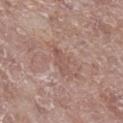  biopsy_status: not biopsied; imaged during a skin examination
  image:
    source: total-body photography crop
    field_of_view_mm: 15
  patient:
    sex: female
    age_approx: 75
  lighting: white-light
  site: right lower leg
  lesion_size:
    long_diameter_mm_approx: 3.5